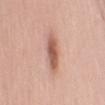Imaged during a routine full-body skin examination; the lesion was not biopsied and no histopathology is available.
The total-body-photography lesion software estimated a shape-asymmetry score of about 0.15 (0 = symmetric). It also reported a nevus-likeness score of about 90/100 and a detector confidence of about 100 out of 100 that the crop contains a lesion.
A close-up tile cropped from a whole-body skin photograph, about 15 mm across.
The subject is a female aged 63–67.
This is a white-light tile.